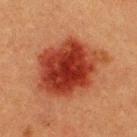Captured during whole-body skin photography for melanoma surveillance; the lesion was not biopsied. About 8.5 mm across. The tile uses cross-polarized illumination. The lesion is located on the upper back. A region of skin cropped from a whole-body photographic capture, roughly 15 mm wide. A male patient approximately 40 years of age. Automated tile analysis of the lesion measured a classifier nevus-likeness of about 100/100.